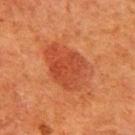<tbp_lesion>
<biopsy_status>not biopsied; imaged during a skin examination</biopsy_status>
<site>right upper arm</site>
<lesion_size>
  <long_diameter_mm_approx>6.0</long_diameter_mm_approx>
</lesion_size>
<patient>
  <sex>female</sex>
  <age_approx>50</age_approx>
</patient>
<image>
  <source>total-body photography crop</source>
  <field_of_view_mm>15</field_of_view_mm>
</image>
<lighting>cross-polarized</lighting>
<automated_metrics>
  <area_mm2_approx>21.0</area_mm2_approx>
  <eccentricity>0.75</eccentricity>
  <shape_asymmetry>0.2</shape_asymmetry>
  <cielab_L>40</cielab_L>
  <cielab_a>29</cielab_a>
  <cielab_b>34</cielab_b>
  <vs_skin_darker_L>8.0</vs_skin_darker_L>
  <vs_skin_contrast_norm>6.5</vs_skin_contrast_norm>
  <border_irregularity_0_10>2.5</border_irregularity_0_10>
  <color_variation_0_10>3.5</color_variation_0_10>
  <peripheral_color_asymmetry>1.5</peripheral_color_asymmetry>
  <nevus_likeness_0_100>95</nevus_likeness_0_100>
  <lesion_detection_confidence_0_100>100</lesion_detection_confidence_0_100>
</automated_metrics>
</tbp_lesion>